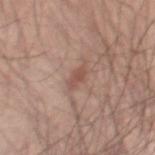This lesion was catalogued during total-body skin photography and was not selected for biopsy.
A lesion tile, about 15 mm wide, cut from a 3D total-body photograph.
From the right thigh.
A male patient aged 43–47.